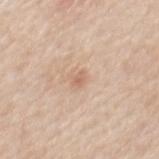Imaged during a routine full-body skin examination; the lesion was not biopsied and no histopathology is available.
A 15 mm close-up tile from a total-body photography series done for melanoma screening.
A male patient, aged approximately 60.
Longest diameter approximately 1.5 mm.
On the back.